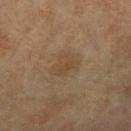Captured during whole-body skin photography for melanoma surveillance; the lesion was not biopsied. Measured at roughly 3.5 mm in maximum diameter. The lesion is located on the leg. The subject is a female aged approximately 70. A 15 mm close-up extracted from a 3D total-body photography capture. Automated tile analysis of the lesion measured a footprint of about 6.5 mm², an eccentricity of roughly 0.65, and a symmetry-axis asymmetry near 0.25. It also reported about 5 CIELAB-L* units darker than the surrounding skin and a normalized lesion–skin contrast near 5.